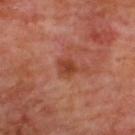Recorded during total-body skin imaging; not selected for excision or biopsy.
Captured under cross-polarized illumination.
From the upper back.
A male subject in their mid-60s.
Approximately 2.5 mm at its widest.
A 15 mm crop from a total-body photograph taken for skin-cancer surveillance.
Automated image analysis of the tile measured a mean CIELAB color near L≈41 a*≈28 b*≈32, roughly 8 lightness units darker than nearby skin, and a normalized border contrast of about 7.5. The analysis additionally found a border-irregularity index near 2.5/10 and a peripheral color-asymmetry measure near 0.5. The analysis additionally found an automated nevus-likeness rating near 30 out of 100 and a lesion-detection confidence of about 100/100.